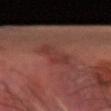biopsy status: no biopsy performed (imaged during a skin exam)
diameter: ≈4 mm
illumination: cross-polarized
anatomic site: the right arm
patient: male, aged approximately 60
automated lesion analysis: an area of roughly 6 mm² and a shape-asymmetry score of about 0.3 (0 = symmetric); border irregularity of about 4 on a 0–10 scale, a within-lesion color-variation index near 2/10, and radial color variation of about 0.5; a classifier nevus-likeness of about 0/100
image: ~15 mm crop, total-body skin-cancer survey